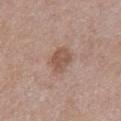The lesion was photographed on a routine skin check and not biopsied; there is no pathology result.
Located on the chest.
A roughly 15 mm field-of-view crop from a total-body skin photograph.
Longest diameter approximately 3.5 mm.
Captured under white-light illumination.
A male patient, about 55 years old.
An algorithmic analysis of the crop reported about 9 CIELAB-L* units darker than the surrounding skin and a lesion-to-skin contrast of about 7 (normalized; higher = more distinct). The software also gave a within-lesion color-variation index near 2.5/10. And it measured a classifier nevus-likeness of about 10/100 and lesion-presence confidence of about 100/100.